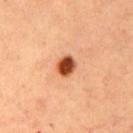Part of a total-body skin-imaging series; this lesion was reviewed on a skin check and was not flagged for biopsy.
About 2.5 mm across.
A lesion tile, about 15 mm wide, cut from a 3D total-body photograph.
This is a cross-polarized tile.
The lesion is located on the mid back.
The patient is a male in their mid-60s.